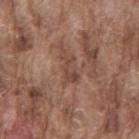workup — imaged on a skin check; not biopsied | acquisition — ~15 mm tile from a whole-body skin photo | subject — male, roughly 75 years of age | illumination — white-light | anatomic site — the back | automated metrics — an area of roughly 4 mm², an outline eccentricity of about 0.9 (0 = round, 1 = elongated), and two-axis asymmetry of about 0.5; a lesion–skin lightness drop of about 8 and a normalized border contrast of about 6; a border-irregularity rating of about 7.5/10, a within-lesion color-variation index near 2/10, and radial color variation of about 0.5; a classifier nevus-likeness of about 0/100 and a lesion-detection confidence of about 75/100.